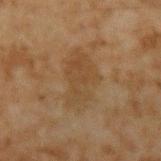biopsy status: no biopsy performed (imaged during a skin exam)
patient: male, roughly 45 years of age
lighting: cross-polarized
image-analysis metrics: a lesion area of about 16 mm² and an outline eccentricity of about 0.85 (0 = round, 1 = elongated)
lesion diameter: ~6 mm (longest diameter)
image: ~15 mm crop, total-body skin-cancer survey
body site: the left upper arm